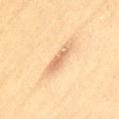Assessment:
This lesion was catalogued during total-body skin photography and was not selected for biopsy.
Image and clinical context:
An algorithmic analysis of the crop reported an average lesion color of about L≈61 a*≈17 b*≈34 (CIELAB). The analysis additionally found a border-irregularity index near 2.5/10, internal color variation of about 2 on a 0–10 scale, and a peripheral color-asymmetry measure near 0.5. A region of skin cropped from a whole-body photographic capture, roughly 15 mm wide. About 4.5 mm across. Captured under cross-polarized illumination. A female patient approximately 40 years of age. The lesion is located on the lower back.